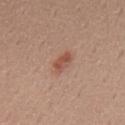biopsy status: imaged on a skin check; not biopsied
location: the mid back
patient: male, aged around 55
imaging modality: ~15 mm tile from a whole-body skin photo
diameter: ≈3 mm